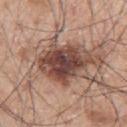A male patient aged around 70.
The recorded lesion diameter is about 5.5 mm.
The lesion is on the left arm.
A region of skin cropped from a whole-body photographic capture, roughly 15 mm wide.